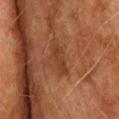Q: Was a biopsy performed?
A: no biopsy performed (imaged during a skin exam)